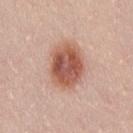biopsy status=catalogued during a skin exam; not biopsied
lighting=white-light illumination
lesion diameter=~5 mm (longest diameter)
site=the lower back
subject=female, aged around 25
image source=total-body-photography crop, ~15 mm field of view